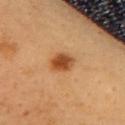Q: Was a biopsy performed?
A: no biopsy performed (imaged during a skin exam)
Q: How was this image acquired?
A: 15 mm crop, total-body photography
Q: What lighting was used for the tile?
A: cross-polarized
Q: What is the anatomic site?
A: the chest
Q: Patient demographics?
A: female, about 45 years old
Q: How large is the lesion?
A: ≈3 mm
Q: What did automated image analysis measure?
A: an average lesion color of about L≈49 a*≈26 b*≈40 (CIELAB) and about 14 CIELAB-L* units darker than the surrounding skin; an automated nevus-likeness rating near 100 out of 100 and a lesion-detection confidence of about 100/100